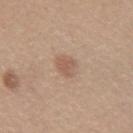Assessment: Imaged during a routine full-body skin examination; the lesion was not biopsied and no histopathology is available. Image and clinical context: A female subject aged 28–32. On the right forearm. A region of skin cropped from a whole-body photographic capture, roughly 15 mm wide. Approximately 3 mm at its widest. Captured under white-light illumination.